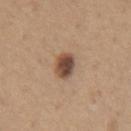Imaged during a routine full-body skin examination; the lesion was not biopsied and no histopathology is available.
The lesion-visualizer software estimated a border-irregularity rating of about 2/10 and peripheral color asymmetry of about 2. The software also gave a lesion-detection confidence of about 100/100.
A male patient, aged around 65.
The lesion is on the chest.
A close-up tile cropped from a whole-body skin photograph, about 15 mm across.
The tile uses white-light illumination.